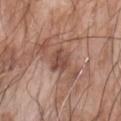{"biopsy_status": "not biopsied; imaged during a skin examination", "image": {"source": "total-body photography crop", "field_of_view_mm": 15}, "lighting": "white-light", "lesion_size": {"long_diameter_mm_approx": 3.0}, "patient": {"sex": "male", "age_approx": 60}, "site": "left forearm"}